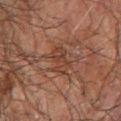follow-up: no biopsy performed (imaged during a skin exam)
subject: male, aged 68–72
anatomic site: the right forearm
acquisition: ~15 mm crop, total-body skin-cancer survey
tile lighting: cross-polarized illumination
TBP lesion metrics: a lesion area of about 5.5 mm², an eccentricity of roughly 0.75, and a shape-asymmetry score of about 0.25 (0 = symmetric)